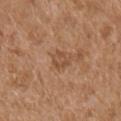Part of a total-body skin-imaging series; this lesion was reviewed on a skin check and was not flagged for biopsy. Captured under white-light illumination. Approximately 2.5 mm at its widest. A lesion tile, about 15 mm wide, cut from a 3D total-body photograph. The total-body-photography lesion software estimated a color-variation rating of about 3/10. The analysis additionally found a lesion-detection confidence of about 100/100. From the right upper arm. The patient is a male aged approximately 75.